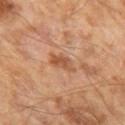Q: Is there a histopathology result?
A: catalogued during a skin exam; not biopsied
Q: Who is the patient?
A: male, approximately 65 years of age
Q: How was the tile lit?
A: cross-polarized illumination
Q: How large is the lesion?
A: ~3 mm (longest diameter)
Q: What kind of image is this?
A: ~15 mm crop, total-body skin-cancer survey